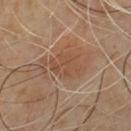{"site": "front of the torso", "image": {"source": "total-body photography crop", "field_of_view_mm": 15}, "patient": {"sex": "male", "age_approx": 45}}